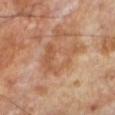Assessment:
Part of a total-body skin-imaging series; this lesion was reviewed on a skin check and was not flagged for biopsy.
Context:
The lesion is located on the leg. The tile uses cross-polarized illumination. Automated image analysis of the tile measured roughly 7 lightness units darker than nearby skin and a lesion-to-skin contrast of about 5.5 (normalized; higher = more distinct). The software also gave internal color variation of about 5.5 on a 0–10 scale and a peripheral color-asymmetry measure near 1.5. A 15 mm close-up extracted from a 3D total-body photography capture. The patient is a male roughly 70 years of age. About 4.5 mm across.